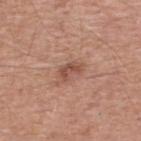Impression: Imaged during a routine full-body skin examination; the lesion was not biopsied and no histopathology is available. Clinical summary: The recorded lesion diameter is about 3 mm. A male subject about 55 years old. This is a white-light tile. A 15 mm close-up extracted from a 3D total-body photography capture. Located on the upper back.